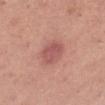Assessment: Recorded during total-body skin imaging; not selected for excision or biopsy. Image and clinical context: Automated image analysis of the tile measured an area of roughly 9 mm², a shape eccentricity near 0.55, and a shape-asymmetry score of about 0.15 (0 = symmetric). It also reported a mean CIELAB color near L≈55 a*≈27 b*≈24 and a lesion-to-skin contrast of about 6.5 (normalized; higher = more distinct). It also reported a nevus-likeness score of about 75/100 and lesion-presence confidence of about 100/100. Captured under white-light illumination. Measured at roughly 3.5 mm in maximum diameter. Cropped from a whole-body photographic skin survey; the tile spans about 15 mm. A female patient, aged 38 to 42. On the leg.